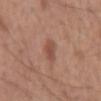Imaged during a routine full-body skin examination; the lesion was not biopsied and no histopathology is available.
A male subject aged 53–57.
A region of skin cropped from a whole-body photographic capture, roughly 15 mm wide.
From the mid back.
About 3 mm across.
The tile uses white-light illumination.